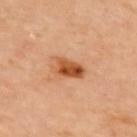image source: ~15 mm tile from a whole-body skin photo, image-analysis metrics: a color-variation rating of about 6.5/10 and a peripheral color-asymmetry measure near 2.5; a classifier nevus-likeness of about 95/100, anatomic site: the back, lesion diameter: ~4 mm (longest diameter).